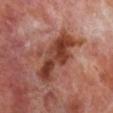Captured during whole-body skin photography for melanoma surveillance; the lesion was not biopsied.
A male patient roughly 70 years of age.
The total-body-photography lesion software estimated a nevus-likeness score of about 0/100 and a detector confidence of about 100 out of 100 that the crop contains a lesion.
Located on the right lower leg.
Captured under cross-polarized illumination.
A 15 mm crop from a total-body photograph taken for skin-cancer surveillance.